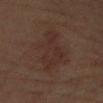The tile uses cross-polarized illumination. An algorithmic analysis of the crop reported an average lesion color of about L≈25 a*≈14 b*≈18 (CIELAB), a lesion–skin lightness drop of about 4, and a normalized lesion–skin contrast near 4.5. And it measured an automated nevus-likeness rating near 5 out of 100. On the right upper arm. The lesion's longest dimension is about 6 mm. A female patient aged 68–72. A 15 mm close-up tile from a total-body photography series done for melanoma screening.